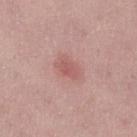Clinical impression:
The lesion was photographed on a routine skin check and not biopsied; there is no pathology result.
Context:
Measured at roughly 3 mm in maximum diameter. A female subject aged around 50. The total-body-photography lesion software estimated an area of roughly 4.5 mm², an outline eccentricity of about 0.8 (0 = round, 1 = elongated), and a shape-asymmetry score of about 0.3 (0 = symmetric). The analysis additionally found a mean CIELAB color near L≈56 a*≈25 b*≈23, a lesion–skin lightness drop of about 8, and a lesion-to-skin contrast of about 5.5 (normalized; higher = more distinct). The software also gave a border-irregularity rating of about 3/10, a color-variation rating of about 1.5/10, and peripheral color asymmetry of about 0.5. Captured under white-light illumination. On the right lower leg. Cropped from a whole-body photographic skin survey; the tile spans about 15 mm.